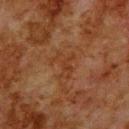Imaged during a routine full-body skin examination; the lesion was not biopsied and no histopathology is available. A male subject, in their 80s. On the upper back. This image is a 15 mm lesion crop taken from a total-body photograph.